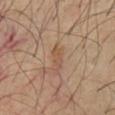| feature | finding |
|---|---|
| follow-up | total-body-photography surveillance lesion; no biopsy |
| image source | ~15 mm crop, total-body skin-cancer survey |
| subject | in their mid- to late 60s |
| location | the front of the torso |